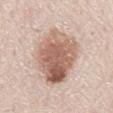The lesion was photographed on a routine skin check and not biopsied; there is no pathology result. A region of skin cropped from a whole-body photographic capture, roughly 15 mm wide. Approximately 9 mm at its widest. From the mid back. The subject is a male aged approximately 80. Imaged with white-light lighting.